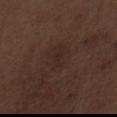Impression:
Imaged during a routine full-body skin examination; the lesion was not biopsied and no histopathology is available.
Image and clinical context:
A 15 mm crop from a total-body photograph taken for skin-cancer surveillance. Automated image analysis of the tile measured an automated nevus-likeness rating near 5 out of 100. A male subject, aged approximately 70. The lesion is on the abdomen.